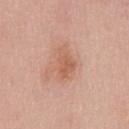biopsy_status: not biopsied; imaged during a skin examination
lighting: white-light
image:
  source: total-body photography crop
  field_of_view_mm: 15
lesion_size:
  long_diameter_mm_approx: 4.5
patient:
  sex: male
  age_approx: 55
automated_metrics:
  vs_skin_contrast_norm: 6.0
  border_irregularity_0_10: 3.0
  color_variation_0_10: 3.0
  peripheral_color_asymmetry: 1.0
  lesion_detection_confidence_0_100: 100
site: chest